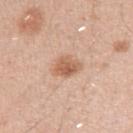Impression:
The lesion was tiled from a total-body skin photograph and was not biopsied.
Clinical summary:
Located on the left upper arm. A male patient, in their 30s. The lesion's longest dimension is about 3.5 mm. This image is a 15 mm lesion crop taken from a total-body photograph.